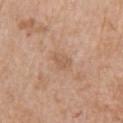Assessment: Captured during whole-body skin photography for melanoma surveillance; the lesion was not biopsied. Image and clinical context: A 15 mm crop from a total-body photograph taken for skin-cancer surveillance. On the right upper arm. The tile uses white-light illumination. The subject is a female in their mid- to late 50s. Approximately 2.5 mm at its widest.